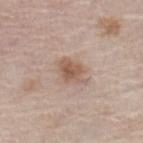<case>
<lighting>white-light</lighting>
<image>
  <source>total-body photography crop</source>
  <field_of_view_mm>15</field_of_view_mm>
</image>
<automated_metrics>
  <vs_skin_darker_L>10.0</vs_skin_darker_L>
  <vs_skin_contrast_norm>7.5</vs_skin_contrast_norm>
  <border_irregularity_0_10>2.5</border_irregularity_0_10>
  <color_variation_0_10>4.0</color_variation_0_10>
  <peripheral_color_asymmetry>1.5</peripheral_color_asymmetry>
  <nevus_likeness_0_100>80</nevus_likeness_0_100>
  <lesion_detection_confidence_0_100>100</lesion_detection_confidence_0_100>
</automated_metrics>
<patient>
  <sex>female</sex>
  <age_approx>65</age_approx>
</patient>
<site>left lower leg</site>
</case>